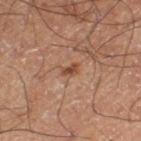The lesion was photographed on a routine skin check and not biopsied; there is no pathology result. The lesion is located on the left thigh. A close-up tile cropped from a whole-body skin photograph, about 15 mm across. This is a cross-polarized tile. A male subject, roughly 60 years of age. The lesion's longest dimension is about 2 mm.